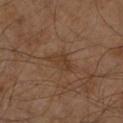Q: What kind of image is this?
A: ~15 mm crop, total-body skin-cancer survey
Q: Who is the patient?
A: male, aged approximately 60
Q: Lesion size?
A: ~3.5 mm (longest diameter)
Q: How was the tile lit?
A: cross-polarized
Q: Where on the body is the lesion?
A: the leg
Q: What did automated image analysis measure?
A: a footprint of about 4.5 mm², an eccentricity of roughly 0.8, and a shape-asymmetry score of about 0.45 (0 = symmetric); a classifier nevus-likeness of about 0/100 and lesion-presence confidence of about 100/100